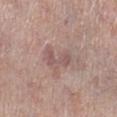workup = total-body-photography surveillance lesion; no biopsy
patient = female, aged approximately 70
anatomic site = the leg
image source = ~15 mm crop, total-body skin-cancer survey